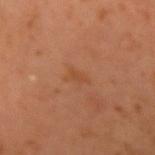No biopsy was performed on this lesion — it was imaged during a full skin examination and was not determined to be concerning.
On the right upper arm.
Captured under cross-polarized illumination.
A male patient, aged 58 to 62.
A 15 mm close-up extracted from a 3D total-body photography capture.